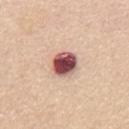  biopsy_status: not biopsied; imaged during a skin examination
  site: mid back
  image:
    source: total-body photography crop
    field_of_view_mm: 15
  patient:
    sex: female
    age_approx: 65